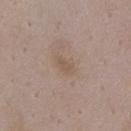Findings:
* biopsy status · catalogued during a skin exam; not biopsied
* subject · female, about 35 years old
* imaging modality · total-body-photography crop, ~15 mm field of view
* automated metrics · a lesion area of about 3.5 mm² and an outline eccentricity of about 0.8 (0 = round, 1 = elongated); a color-variation rating of about 1/10; an automated nevus-likeness rating near 0 out of 100 and a detector confidence of about 100 out of 100 that the crop contains a lesion
* illumination · white-light
* site · the front of the torso